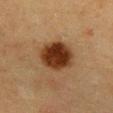Assessment:
Recorded during total-body skin imaging; not selected for excision or biopsy.
Image and clinical context:
An algorithmic analysis of the crop reported a lesion color around L≈29 a*≈19 b*≈28 in CIELAB and a lesion–skin lightness drop of about 15. The analysis additionally found a classifier nevus-likeness of about 100/100 and a lesion-detection confidence of about 100/100. About 5 mm across. This image is a 15 mm lesion crop taken from a total-body photograph. Captured under cross-polarized illumination. On the abdomen. A female subject in their mid-50s.